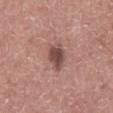This lesion was catalogued during total-body skin photography and was not selected for biopsy. Measured at roughly 3.5 mm in maximum diameter. A male subject, in their 60s. From the abdomen. A 15 mm crop from a total-body photograph taken for skin-cancer surveillance.